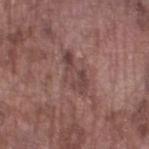notes: total-body-photography surveillance lesion; no biopsy
subject: male, in their mid- to late 70s
image: total-body-photography crop, ~15 mm field of view
body site: the left thigh
diameter: about 5 mm